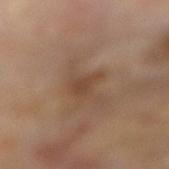Notes:
– notes: no biopsy performed (imaged during a skin exam)
– acquisition: ~15 mm tile from a whole-body skin photo
– lighting: cross-polarized
– automated metrics: an average lesion color of about L≈44 a*≈18 b*≈29 (CIELAB) and a normalized lesion–skin contrast near 6; a classifier nevus-likeness of about 0/100 and a lesion-detection confidence of about 100/100
– location: the right forearm
– patient: female, aged 73–77
– lesion diameter: ≈3 mm